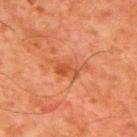Impression:
The lesion was photographed on a routine skin check and not biopsied; there is no pathology result.
Image and clinical context:
Located on the mid back. Longest diameter approximately 4.5 mm. A male subject aged approximately 80. A 15 mm crop from a total-body photograph taken for skin-cancer surveillance. Imaged with cross-polarized lighting.